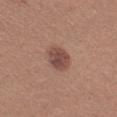Part of a total-body skin-imaging series; this lesion was reviewed on a skin check and was not flagged for biopsy. Cropped from a whole-body photographic skin survey; the tile spans about 15 mm. The subject is a male about 25 years old. From the left upper arm.